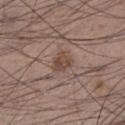{
  "biopsy_status": "not biopsied; imaged during a skin examination",
  "site": "left lower leg",
  "patient": {
    "sex": "male",
    "age_approx": 35
  },
  "lighting": "white-light",
  "automated_metrics": {
    "color_variation_0_10": 3.0,
    "peripheral_color_asymmetry": 1.0,
    "nevus_likeness_0_100": 70,
    "lesion_detection_confidence_0_100": 100
  },
  "image": {
    "source": "total-body photography crop",
    "field_of_view_mm": 15
  },
  "lesion_size": {
    "long_diameter_mm_approx": 2.5
  }
}